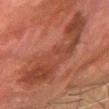Assessment:
No biopsy was performed on this lesion — it was imaged during a full skin examination and was not determined to be concerning.
Context:
The subject is a male aged 78–82. A close-up tile cropped from a whole-body skin photograph, about 15 mm across. The recorded lesion diameter is about 9 mm. The lesion is located on the right forearm.